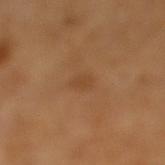{
  "biopsy_status": "not biopsied; imaged during a skin examination",
  "image": {
    "source": "total-body photography crop",
    "field_of_view_mm": 15
  },
  "lighting": "cross-polarized",
  "site": "right lower leg",
  "patient": {
    "sex": "female",
    "age_approx": 55
  },
  "automated_metrics": {
    "area_mm2_approx": 3.0,
    "eccentricity": 0.85,
    "shape_asymmetry": 0.25,
    "vs_skin_darker_L": 6.0
  }
}